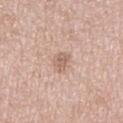Imaged during a routine full-body skin examination; the lesion was not biopsied and no histopathology is available. The subject is a male aged approximately 50. Longest diameter approximately 3 mm. Captured under white-light illumination. A region of skin cropped from a whole-body photographic capture, roughly 15 mm wide. The lesion is located on the right lower leg.